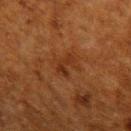| key | value |
|---|---|
| imaging modality | 15 mm crop, total-body photography |
| lighting | cross-polarized illumination |
| site | the arm |
| image-analysis metrics | a lesion color around L≈26 a*≈21 b*≈30 in CIELAB, about 6 CIELAB-L* units darker than the surrounding skin, and a normalized lesion–skin contrast near 6.5; radial color variation of about 0; a classifier nevus-likeness of about 0/100 and a detector confidence of about 100 out of 100 that the crop contains a lesion |
| subject | male, about 60 years old |
| size | ≈3 mm |